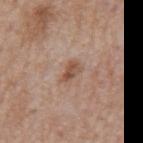follow-up: total-body-photography surveillance lesion; no biopsy | subject: male, aged 63 to 67 | lesion size: ~3 mm (longest diameter) | image: 15 mm crop, total-body photography | location: the back | lighting: white-light | image-analysis metrics: a lesion area of about 3.5 mm² and an outline eccentricity of about 0.85 (0 = round, 1 = elongated).